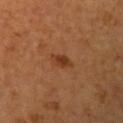Notes:
• biopsy status — no biopsy performed (imaged during a skin exam)
• image-analysis metrics — a shape eccentricity near 0.85; an automated nevus-likeness rating near 90 out of 100 and a lesion-detection confidence of about 100/100
• patient — female, in their 40s
• lighting — cross-polarized
• lesion size — ~3 mm (longest diameter)
• image — 15 mm crop, total-body photography
• body site — the left upper arm